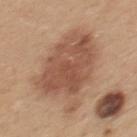- follow-up — no biopsy performed (imaged during a skin exam)
- lesion size — ≈7.5 mm
- automated metrics — an average lesion color of about L≈53 a*≈21 b*≈30 (CIELAB) and a normalized lesion–skin contrast near 8; a border-irregularity rating of about 3.5/10 and a within-lesion color-variation index near 4.5/10
- lighting — white-light illumination
- site — the upper back
- image — 15 mm crop, total-body photography
- patient — female, aged 43–47